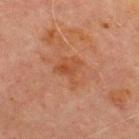illumination = cross-polarized
location = the chest
patient = male, aged 68–72
automated lesion analysis = a border-irregularity index near 5/10, internal color variation of about 4 on a 0–10 scale, and radial color variation of about 1; a classifier nevus-likeness of about 0/100 and a detector confidence of about 100 out of 100 that the crop contains a lesion
image = ~15 mm tile from a whole-body skin photo
lesion diameter = about 3 mm